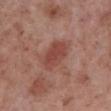Impression:
Imaged during a routine full-body skin examination; the lesion was not biopsied and no histopathology is available.
Image and clinical context:
Located on the right lower leg. A lesion tile, about 15 mm wide, cut from a 3D total-body photograph. This is a white-light tile. The subject is a male in their 70s. The lesion-visualizer software estimated a lesion area of about 9 mm², an eccentricity of roughly 0.7, and a symmetry-axis asymmetry near 0.2. It also reported a lesion color around L≈45 a*≈26 b*≈25 in CIELAB and a normalized lesion–skin contrast near 7. And it measured an automated nevus-likeness rating near 35 out of 100 and lesion-presence confidence of about 100/100.